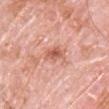Recorded during total-body skin imaging; not selected for excision or biopsy. A 15 mm close-up extracted from a 3D total-body photography capture. The subject is a male aged around 80. Imaged with white-light lighting. From the chest. Measured at roughly 3.5 mm in maximum diameter.